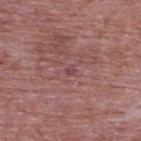The lesion was tiled from a total-body skin photograph and was not biopsied. The tile uses white-light illumination. About 1.5 mm across. A lesion tile, about 15 mm wide, cut from a 3D total-body photograph. A male subject about 75 years old. From the upper back.